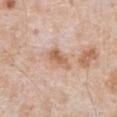A 15 mm close-up extracted from a 3D total-body photography capture. On the front of the torso. Longest diameter approximately 4 mm. A male subject, approximately 65 years of age. Captured under white-light illumination.